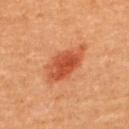The lesion was tiled from a total-body skin photograph and was not biopsied.